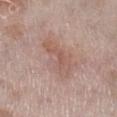Recorded during total-body skin imaging; not selected for excision or biopsy. Located on the leg. A region of skin cropped from a whole-body photographic capture, roughly 15 mm wide. A male patient, approximately 60 years of age.